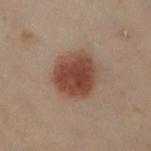<tbp_lesion>
<biopsy_status>not biopsied; imaged during a skin examination</biopsy_status>
<lesion_size>
  <long_diameter_mm_approx>5.0</long_diameter_mm_approx>
</lesion_size>
<automated_metrics>
  <eccentricity>0.25</eccentricity>
  <shape_asymmetry>0.1</shape_asymmetry>
  <border_irregularity_0_10>1.0</border_irregularity_0_10>
  <color_variation_0_10>4.0</color_variation_0_10>
  <peripheral_color_asymmetry>1.0</peripheral_color_asymmetry>
  <lesion_detection_confidence_0_100>100</lesion_detection_confidence_0_100>
</automated_metrics>
<image>
  <source>total-body photography crop</source>
  <field_of_view_mm>15</field_of_view_mm>
</image>
<patient>
  <sex>female</sex>
  <age_approx>60</age_approx>
</patient>
<lighting>cross-polarized</lighting>
<site>left leg</site>
</tbp_lesion>